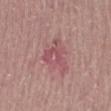Notes:
* notes: catalogued during a skin exam; not biopsied
* body site: the right lower leg
* subject: female, aged 63 to 67
* acquisition: total-body-photography crop, ~15 mm field of view